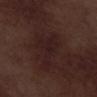The lesion is located on the right lower leg.
Imaged with white-light lighting.
An algorithmic analysis of the crop reported an eccentricity of roughly 0.8 and a symmetry-axis asymmetry near 0.6. And it measured a lesion color around L≈19 a*≈17 b*≈15 in CIELAB, about 4 CIELAB-L* units darker than the surrounding skin, and a normalized lesion–skin contrast near 6.5. It also reported border irregularity of about 7 on a 0–10 scale and internal color variation of about 2 on a 0–10 scale. And it measured a nevus-likeness score of about 0/100 and a detector confidence of about 95 out of 100 that the crop contains a lesion.
A region of skin cropped from a whole-body photographic capture, roughly 15 mm wide.
A male subject, aged 68–72.
Approximately 6 mm at its widest.